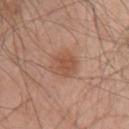Case summary:
– notes · no biopsy performed (imaged during a skin exam)
– patient · male, in their mid-40s
– automated lesion analysis · an area of roughly 9.5 mm², an outline eccentricity of about 0.6 (0 = round, 1 = elongated), and a symmetry-axis asymmetry near 0.2; an automated nevus-likeness rating near 70 out of 100 and lesion-presence confidence of about 100/100
– tile lighting · white-light
– acquisition · ~15 mm tile from a whole-body skin photo
– body site · the left upper arm
– lesion size · ~4 mm (longest diameter)